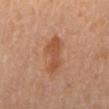{
  "automated_metrics": {
    "shape_asymmetry": 0.35
  },
  "patient": {
    "sex": "male",
    "age_approx": 65
  },
  "image": {
    "source": "total-body photography crop",
    "field_of_view_mm": 15
  },
  "site": "mid back"
}